The subject is a male about 85 years old or older; the lesion is on the head or neck; a 15 mm close-up extracted from a 3D total-body photography capture; the lesion's longest dimension is about 1.5 mm:
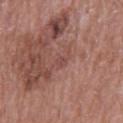Q: What did the biopsy show?
A: a melanoma in situ — a skin cancer